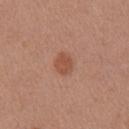Captured during whole-body skin photography for melanoma surveillance; the lesion was not biopsied.
A 15 mm close-up tile from a total-body photography series done for melanoma screening.
Captured under white-light illumination.
A female patient aged around 35.
The lesion's longest dimension is about 2.5 mm.
An algorithmic analysis of the crop reported an eccentricity of roughly 0.6 and a shape-asymmetry score of about 0.1 (0 = symmetric). The analysis additionally found a mean CIELAB color near L≈51 a*≈24 b*≈31, a lesion–skin lightness drop of about 8, and a normalized border contrast of about 6.5. The software also gave a border-irregularity index near 1/10, internal color variation of about 1.5 on a 0–10 scale, and peripheral color asymmetry of about 0.5.
The lesion is on the arm.